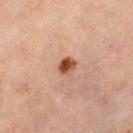The lesion's longest dimension is about 2 mm. Captured under cross-polarized illumination. Automated tile analysis of the lesion measured a footprint of about 3.5 mm², a shape eccentricity near 0.55, and a symmetry-axis asymmetry near 0.15. The analysis additionally found a nevus-likeness score of about 100/100. Located on the left thigh. A 15 mm close-up extracted from a 3D total-body photography capture. A male patient, about 70 years old.